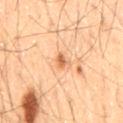| key | value |
|---|---|
| workup | total-body-photography surveillance lesion; no biopsy |
| automated metrics | a mean CIELAB color near L≈64 a*≈26 b*≈41 and a normalized lesion–skin contrast near 7.5; a border-irregularity index near 3/10, a within-lesion color-variation index near 1.5/10, and peripheral color asymmetry of about 0.5 |
| image | total-body-photography crop, ~15 mm field of view |
| subject | male, aged approximately 55 |
| anatomic site | the mid back |